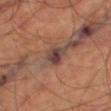No biopsy was performed on this lesion — it was imaged during a full skin examination and was not determined to be concerning.
Located on the right thigh.
Automated tile analysis of the lesion measured a border-irregularity index near 2.5/10, a within-lesion color-variation index near 4.5/10, and radial color variation of about 1.5.
This image is a 15 mm lesion crop taken from a total-body photograph.
The subject is a male aged 68–72.